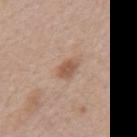Imaged during a routine full-body skin examination; the lesion was not biopsied and no histopathology is available. On the mid back. The lesion-visualizer software estimated an automated nevus-likeness rating near 55 out of 100 and a lesion-detection confidence of about 100/100. The tile uses white-light illumination. The patient is a male in their mid-60s. Cropped from a total-body skin-imaging series; the visible field is about 15 mm. The lesion's longest dimension is about 3 mm.